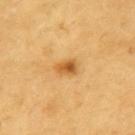follow-up = total-body-photography surveillance lesion; no biopsy | lighting = cross-polarized | subject = male, aged around 60 | image source = ~15 mm crop, total-body skin-cancer survey | site = the left upper arm.